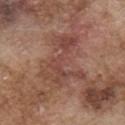| feature | finding |
|---|---|
| notes | no biopsy performed (imaged during a skin exam) |
| location | the chest |
| patient | male, aged approximately 75 |
| lesion diameter | about 7.5 mm |
| tile lighting | white-light |
| image source | ~15 mm crop, total-body skin-cancer survey |